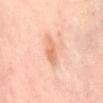This lesion was catalogued during total-body skin photography and was not selected for biopsy.
Automated tile analysis of the lesion measured a footprint of about 5.5 mm², an outline eccentricity of about 0.9 (0 = round, 1 = elongated), and two-axis asymmetry of about 0.25. It also reported a mean CIELAB color near L≈68 a*≈24 b*≈34, a lesion–skin lightness drop of about 9, and a normalized border contrast of about 6.5.
Located on the abdomen.
A female subject roughly 50 years of age.
Captured under cross-polarized illumination.
Cropped from a whole-body photographic skin survey; the tile spans about 15 mm.